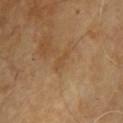Assessment: Captured during whole-body skin photography for melanoma surveillance; the lesion was not biopsied. Acquisition and patient details: A 15 mm close-up tile from a total-body photography series done for melanoma screening. The lesion is located on the chest. A male patient aged around 65.